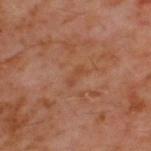The lesion was photographed on a routine skin check and not biopsied; there is no pathology result. The total-body-photography lesion software estimated a footprint of about 2 mm², an outline eccentricity of about 0.95 (0 = round, 1 = elongated), and two-axis asymmetry of about 0.35. It also reported a lesion color around L≈43 a*≈23 b*≈32 in CIELAB, a lesion–skin lightness drop of about 4, and a normalized lesion–skin contrast near 4.5. It also reported a border-irregularity rating of about 4/10, internal color variation of about 0 on a 0–10 scale, and a peripheral color-asymmetry measure near 0. And it measured a nevus-likeness score of about 0/100 and a lesion-detection confidence of about 100/100. A male subject, aged 58–62. A roughly 15 mm field-of-view crop from a total-body skin photograph. The lesion's longest dimension is about 2.5 mm. From the upper back. The tile uses cross-polarized illumination.